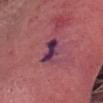Assessment:
Captured during whole-body skin photography for melanoma surveillance; the lesion was not biopsied.
Context:
A close-up tile cropped from a whole-body skin photograph, about 15 mm across. A male subject, in their mid-70s. Located on the head or neck. The recorded lesion diameter is about 4 mm.